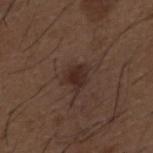* notes — catalogued during a skin exam; not biopsied
* site — the mid back
* diameter — ~3 mm (longest diameter)
* patient — male, roughly 50 years of age
* image source — ~15 mm tile from a whole-body skin photo